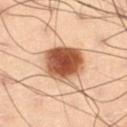follow-up: total-body-photography surveillance lesion; no biopsy
patient: male, aged 53–57
size: ~6 mm (longest diameter)
imaging modality: total-body-photography crop, ~15 mm field of view
TBP lesion metrics: a footprint of about 18 mm², a shape eccentricity near 0.75, and a symmetry-axis asymmetry near 0.15; a mean CIELAB color near L≈44 a*≈20 b*≈29 and a lesion–skin lightness drop of about 18; a detector confidence of about 100 out of 100 that the crop contains a lesion
location: the left thigh
illumination: cross-polarized